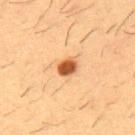Captured during whole-body skin photography for melanoma surveillance; the lesion was not biopsied.
Approximately 2.5 mm at its widest.
From the front of the torso.
A male patient, approximately 55 years of age.
This is a cross-polarized tile.
A 15 mm close-up tile from a total-body photography series done for melanoma screening.